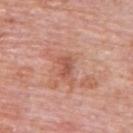The lesion was photographed on a routine skin check and not biopsied; there is no pathology result. A male patient about 80 years old. A 15 mm close-up extracted from a 3D total-body photography capture. The lesion is located on the upper back.